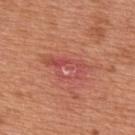  biopsy_status: not biopsied; imaged during a skin examination
  lesion_size:
    long_diameter_mm_approx: 5.5
  patient:
    sex: male
    age_approx: 65
  image:
    source: total-body photography crop
    field_of_view_mm: 15
  lighting: white-light
  site: upper back